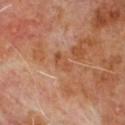<record>
  <lesion_size>
    <long_diameter_mm_approx>2.5</long_diameter_mm_approx>
  </lesion_size>
  <site>chest</site>
  <automated_metrics>
    <area_mm2_approx>3.5</area_mm2_approx>
    <eccentricity>0.85</eccentricity>
    <shape_asymmetry>0.25</shape_asymmetry>
    <cielab_L>49</cielab_L>
    <cielab_a>23</cielab_a>
    <cielab_b>33</cielab_b>
    <vs_skin_contrast_norm>5.5</vs_skin_contrast_norm>
    <border_irregularity_0_10>2.5</border_irregularity_0_10>
    <color_variation_0_10>1.5</color_variation_0_10>
  </automated_metrics>
  <lighting>cross-polarized</lighting>
  <patient>
    <sex>male</sex>
    <age_approx>65</age_approx>
  </patient>
  <image>
    <source>total-body photography crop</source>
    <field_of_view_mm>15</field_of_view_mm>
  </image>
</record>